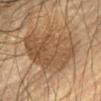This lesion was catalogued during total-body skin photography and was not selected for biopsy. A male patient, in their mid-60s. The lesion is on the front of the torso. Cropped from a total-body skin-imaging series; the visible field is about 15 mm. The tile uses cross-polarized illumination. Approximately 8.5 mm at its widest.